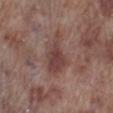Case summary:
- biopsy status · total-body-photography surveillance lesion; no biopsy
- patient · male, approximately 70 years of age
- site · the right lower leg
- image source · 15 mm crop, total-body photography